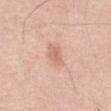<record>
<biopsy_status>not biopsied; imaged during a skin examination</biopsy_status>
<lighting>white-light</lighting>
<image>
  <source>total-body photography crop</source>
  <field_of_view_mm>15</field_of_view_mm>
</image>
<site>abdomen</site>
<patient>
  <sex>male</sex>
  <age_approx>50</age_approx>
</patient>
<lesion_size>
  <long_diameter_mm_approx>3.0</long_diameter_mm_approx>
</lesion_size>
</record>